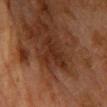Impression:
The lesion was photographed on a routine skin check and not biopsied; there is no pathology result.
Acquisition and patient details:
A female subject in their mid-50s. The recorded lesion diameter is about 5 mm. A 15 mm close-up extracted from a 3D total-body photography capture. From the chest.